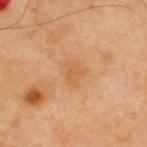workup: catalogued during a skin exam; not biopsied | imaging modality: ~15 mm tile from a whole-body skin photo | size: ~2.5 mm (longest diameter) | location: the upper back | automated metrics: an area of roughly 4 mm², an eccentricity of roughly 0.6, and two-axis asymmetry of about 0.4; a lesion–skin lightness drop of about 6 and a normalized lesion–skin contrast near 5; a classifier nevus-likeness of about 0/100 and a lesion-detection confidence of about 100/100 | subject: male, in their 40s.